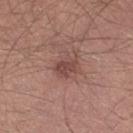Impression:
Part of a total-body skin-imaging series; this lesion was reviewed on a skin check and was not flagged for biopsy.
Background:
An algorithmic analysis of the crop reported a mean CIELAB color near L≈46 a*≈21 b*≈23 and a normalized lesion–skin contrast near 6.5. The software also gave border irregularity of about 2 on a 0–10 scale and peripheral color asymmetry of about 1. The patient is a male aged 28 to 32. Imaged with white-light lighting. A region of skin cropped from a whole-body photographic capture, roughly 15 mm wide. The lesion is on the right thigh. The recorded lesion diameter is about 3 mm.